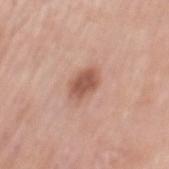A region of skin cropped from a whole-body photographic capture, roughly 15 mm wide. The tile uses white-light illumination. Automated image analysis of the tile measured a lesion area of about 7 mm² and a symmetry-axis asymmetry near 0.2. The software also gave a mean CIELAB color near L≈55 a*≈23 b*≈28 and a lesion–skin lightness drop of about 12. The analysis additionally found a color-variation rating of about 2.5/10 and peripheral color asymmetry of about 0.5. The lesion is located on the mid back. A female patient, aged approximately 60.